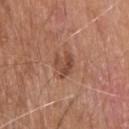follow-up=catalogued during a skin exam; not biopsied | subject=male, aged 78–82 | size=≈3 mm | illumination=white-light illumination | location=the head or neck | image source=~15 mm crop, total-body skin-cancer survey.